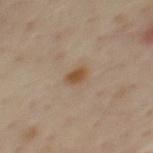Q: Was this lesion biopsied?
A: total-body-photography surveillance lesion; no biopsy
Q: What kind of image is this?
A: ~15 mm tile from a whole-body skin photo
Q: Who is the patient?
A: male, roughly 45 years of age
Q: Lesion size?
A: ≈2.5 mm
Q: What is the anatomic site?
A: the mid back